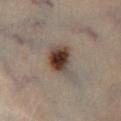{"biopsy_status": "not biopsied; imaged during a skin examination", "patient": {"sex": "male", "age_approx": 55}, "site": "left lower leg", "image": {"source": "total-body photography crop", "field_of_view_mm": 15}, "lesion_size": {"long_diameter_mm_approx": 4.5}}